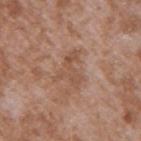Located on the right upper arm. Imaged with white-light lighting. A region of skin cropped from a whole-body photographic capture, roughly 15 mm wide. The subject is a male aged approximately 45. Automated image analysis of the tile measured a lesion area of about 7.5 mm², an outline eccentricity of about 0.7 (0 = round, 1 = elongated), and a symmetry-axis asymmetry near 0.75. The recorded lesion diameter is about 4 mm.